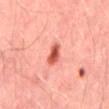biopsy status: catalogued during a skin exam; not biopsied | subject: male, about 45 years old | anatomic site: the lower back | image source: 15 mm crop, total-body photography | illumination: cross-polarized illumination | lesion diameter: ~2.5 mm (longest diameter).